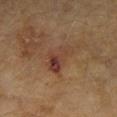This lesion was catalogued during total-body skin photography and was not selected for biopsy. Cropped from a whole-body photographic skin survey; the tile spans about 15 mm. The total-body-photography lesion software estimated a lesion color around L≈35 a*≈19 b*≈25 in CIELAB and a lesion–skin lightness drop of about 7. The software also gave a border-irregularity rating of about 5/10 and internal color variation of about 8.5 on a 0–10 scale. It also reported a lesion-detection confidence of about 100/100. A female patient, aged approximately 60. From the left forearm. About 4 mm across.